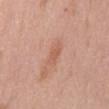On the mid back.
Cropped from a total-body skin-imaging series; the visible field is about 15 mm.
Automated tile analysis of the lesion measured an average lesion color of about L≈59 a*≈23 b*≈31 (CIELAB) and about 7 CIELAB-L* units darker than the surrounding skin. And it measured border irregularity of about 2.5 on a 0–10 scale, a color-variation rating of about 1.5/10, and radial color variation of about 0.5.
Imaged with white-light lighting.
A male patient, aged 68–72.